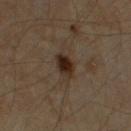No biopsy was performed on this lesion — it was imaged during a full skin examination and was not determined to be concerning.
The subject is a male aged 58–62.
A 15 mm close-up extracted from a 3D total-body photography capture.
Captured under cross-polarized illumination.
From the right upper arm.